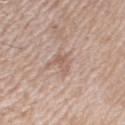biopsy status: no biopsy performed (imaged during a skin exam)
size: about 2.5 mm
image: ~15 mm crop, total-body skin-cancer survey
body site: the right upper arm
tile lighting: white-light
patient: male, roughly 60 years of age
automated lesion analysis: a lesion-to-skin contrast of about 5.5 (normalized; higher = more distinct); a border-irregularity index near 4.5/10, internal color variation of about 3 on a 0–10 scale, and a peripheral color-asymmetry measure near 1